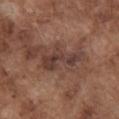Q: Was this lesion biopsied?
A: catalogued during a skin exam; not biopsied
Q: Where on the body is the lesion?
A: the front of the torso
Q: Lesion size?
A: ~5.5 mm (longest diameter)
Q: What kind of image is this?
A: total-body-photography crop, ~15 mm field of view
Q: Who is the patient?
A: male, aged 73 to 77
Q: What lighting was used for the tile?
A: white-light